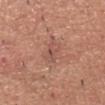This lesion was catalogued during total-body skin photography and was not selected for biopsy. About 3 mm across. Imaged with white-light lighting. A 15 mm close-up extracted from a 3D total-body photography capture. On the head or neck. Automated tile analysis of the lesion measured a lesion area of about 4.5 mm² and a shape-asymmetry score of about 0.2 (0 = symmetric). The analysis additionally found a lesion color around L≈52 a*≈23 b*≈26 in CIELAB, a lesion–skin lightness drop of about 7, and a normalized border contrast of about 5.5. The software also gave border irregularity of about 2.5 on a 0–10 scale and radial color variation of about 1.5. A male patient, aged 53–57.